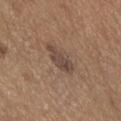biopsy status: catalogued during a skin exam; not biopsied
patient: male, about 65 years old
image source: ~15 mm crop, total-body skin-cancer survey
anatomic site: the abdomen
lighting: white-light
size: about 4.5 mm